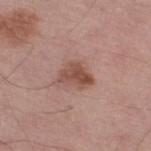notes: no biopsy performed (imaged during a skin exam) | tile lighting: white-light | lesion diameter: ~4 mm (longest diameter) | anatomic site: the leg | patient: male, aged around 70 | imaging modality: ~15 mm crop, total-body skin-cancer survey | TBP lesion metrics: a mean CIELAB color near L≈50 a*≈22 b*≈26, about 11 CIELAB-L* units darker than the surrounding skin, and a normalized border contrast of about 8; a within-lesion color-variation index near 4.5/10 and peripheral color asymmetry of about 2; an automated nevus-likeness rating near 45 out of 100 and a detector confidence of about 100 out of 100 that the crop contains a lesion.